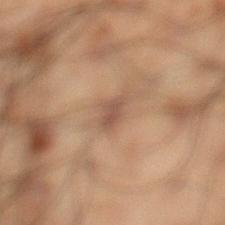Notes:
* workup: imaged on a skin check; not biopsied
* image source: 15 mm crop, total-body photography
* subject: male, roughly 50 years of age
* body site: the left lower leg